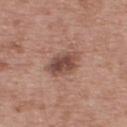The lesion was photographed on a routine skin check and not biopsied; there is no pathology result.
Imaged with white-light lighting.
A close-up tile cropped from a whole-body skin photograph, about 15 mm across.
On the upper back.
The subject is a male aged 63 to 67.
The total-body-photography lesion software estimated an eccentricity of roughly 0.75. The analysis additionally found border irregularity of about 2 on a 0–10 scale, internal color variation of about 4.5 on a 0–10 scale, and a peripheral color-asymmetry measure near 1.5. The software also gave a lesion-detection confidence of about 100/100.